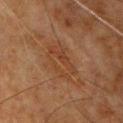Context: Captured under cross-polarized illumination. A male subject, aged approximately 60. Located on the chest. Longest diameter approximately 5 mm. This image is a 15 mm lesion crop taken from a total-body photograph. The total-body-photography lesion software estimated an area of roughly 11 mm², a shape eccentricity near 0.85, and two-axis asymmetry of about 0.25. It also reported roughly 5 lightness units darker than nearby skin and a normalized border contrast of about 5.